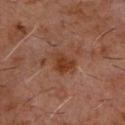notes = no biopsy performed (imaged during a skin exam); lesion size = about 3 mm; tile lighting = cross-polarized illumination; imaging modality = total-body-photography crop, ~15 mm field of view; location = the front of the torso; patient = male, aged 58 to 62.